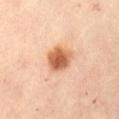workup = no biopsy performed (imaged during a skin exam)
patient = female, aged approximately 60
illumination = cross-polarized
automated metrics = an average lesion color of about L≈64 a*≈25 b*≈39 (CIELAB) and about 16 CIELAB-L* units darker than the surrounding skin; a color-variation rating of about 6/10 and a peripheral color-asymmetry measure near 1.5; an automated nevus-likeness rating near 100 out of 100
acquisition = 15 mm crop, total-body photography
anatomic site = the front of the torso